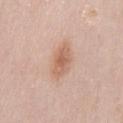Q: Lesion size?
A: about 4.5 mm
Q: Lesion location?
A: the abdomen
Q: How was this image acquired?
A: ~15 mm crop, total-body skin-cancer survey
Q: Who is the patient?
A: male, aged 63–67
Q: Illumination type?
A: white-light illumination